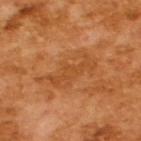A male patient aged 63 to 67.
This is a cross-polarized tile.
Cropped from a whole-body photographic skin survey; the tile spans about 15 mm.
About 6.5 mm across.